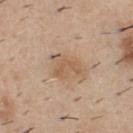<tbp_lesion>
  <image>
    <source>total-body photography crop</source>
    <field_of_view_mm>15</field_of_view_mm>
  </image>
  <site>chest</site>
  <lighting>white-light</lighting>
  <patient>
    <sex>male</sex>
    <age_approx>40</age_approx>
  </patient>
  <lesion_size>
    <long_diameter_mm_approx>4.0</long_diameter_mm_approx>
  </lesion_size>
</tbp_lesion>